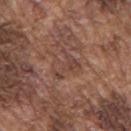follow-up: catalogued during a skin exam; not biopsied
TBP lesion metrics: a lesion–skin lightness drop of about 6
patient: male, approximately 75 years of age
size: ≈4 mm
tile lighting: white-light
image: ~15 mm tile from a whole-body skin photo
location: the upper back